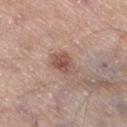Imaged with white-light lighting.
Approximately 3.5 mm at its widest.
A 15 mm close-up extracted from a 3D total-body photography capture.
The lesion is located on the right thigh.
A male subject approximately 80 years of age.